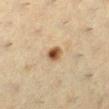• biopsy status: no biopsy performed (imaged during a skin exam)
• body site: the left lower leg
• imaging modality: ~15 mm tile from a whole-body skin photo
• subject: female, about 40 years old